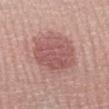biopsy status: no biopsy performed (imaged during a skin exam) | patient: male, roughly 70 years of age | imaging modality: ~15 mm tile from a whole-body skin photo | diameter: ~6 mm (longest diameter) | anatomic site: the right lower leg | tile lighting: white-light.